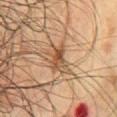The lesion was photographed on a routine skin check and not biopsied; there is no pathology result. The tile uses cross-polarized illumination. A male patient aged approximately 70. Located on the chest. A lesion tile, about 15 mm wide, cut from a 3D total-body photograph.